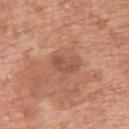| feature | finding |
|---|---|
| biopsy status | imaged on a skin check; not biopsied |
| illumination | white-light illumination |
| site | the back |
| image source | 15 mm crop, total-body photography |
| patient | male, roughly 75 years of age |